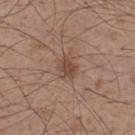| key | value |
|---|---|
| notes | imaged on a skin check; not biopsied |
| subject | male, roughly 50 years of age |
| image source | total-body-photography crop, ~15 mm field of view |
| site | the upper back |